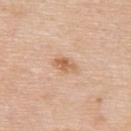Assessment:
This lesion was catalogued during total-body skin photography and was not selected for biopsy.
Image and clinical context:
Cropped from a whole-body photographic skin survey; the tile spans about 15 mm. The lesion is on the upper back. Measured at roughly 3 mm in maximum diameter. The patient is a male roughly 60 years of age.